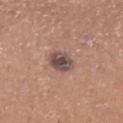workup: no biopsy performed (imaged during a skin exam).